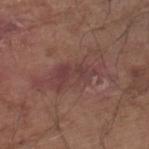Image and clinical context: Imaged with white-light lighting. The recorded lesion diameter is about 8.5 mm. A region of skin cropped from a whole-body photographic capture, roughly 15 mm wide. A male patient aged 78–82. From the right lower leg.